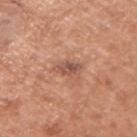Context:
The recorded lesion diameter is about 3 mm. Automated tile analysis of the lesion measured a footprint of about 4.5 mm² and two-axis asymmetry of about 0.25. The software also gave a mean CIELAB color near L≈53 a*≈23 b*≈28, about 10 CIELAB-L* units darker than the surrounding skin, and a normalized border contrast of about 7.5. A roughly 15 mm field-of-view crop from a total-body skin photograph. A male subject roughly 55 years of age. From the right upper arm.